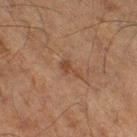follow-up: catalogued during a skin exam; not biopsied
patient: male, about 70 years old
automated metrics: a footprint of about 3 mm² and a shape eccentricity near 0.85; a border-irregularity index near 5.5/10 and peripheral color asymmetry of about 0; a lesion-detection confidence of about 100/100
lesion size: ≈2.5 mm
tile lighting: cross-polarized
location: the right thigh
imaging modality: ~15 mm tile from a whole-body skin photo